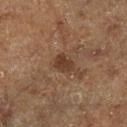Recorded during total-body skin imaging; not selected for excision or biopsy.
This image is a 15 mm lesion crop taken from a total-body photograph.
About 2.5 mm across.
The lesion is located on the left lower leg.
The lesion-visualizer software estimated a lesion area of about 4 mm², an outline eccentricity of about 0.7 (0 = round, 1 = elongated), and a shape-asymmetry score of about 0.25 (0 = symmetric).
A male patient aged around 75.